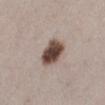Imaged during a routine full-body skin examination; the lesion was not biopsied and no histopathology is available. The lesion is located on the left lower leg. Cropped from a total-body skin-imaging series; the visible field is about 15 mm. A female patient approximately 40 years of age.